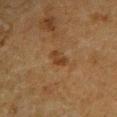Recorded during total-body skin imaging; not selected for excision or biopsy. Approximately 2.5 mm at its widest. Cropped from a total-body skin-imaging series; the visible field is about 15 mm. A male patient roughly 60 years of age. Captured under cross-polarized illumination. The lesion is on the leg.